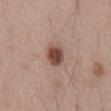Q: Is there a histopathology result?
A: imaged on a skin check; not biopsied
Q: What is the lesion's diameter?
A: about 3 mm
Q: What lighting was used for the tile?
A: white-light illumination
Q: Lesion location?
A: the back
Q: What are the patient's age and sex?
A: male, aged around 50
Q: What kind of image is this?
A: ~15 mm tile from a whole-body skin photo